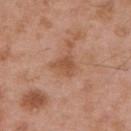<case>
<image>
  <source>total-body photography crop</source>
  <field_of_view_mm>15</field_of_view_mm>
</image>
<site>back</site>
<patient>
  <sex>male</sex>
  <age_approx>55</age_approx>
</patient>
<lighting>white-light</lighting>
</case>